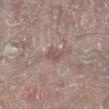{"biopsy_status": "not biopsied; imaged during a skin examination", "site": "leg", "patient": {"sex": "male", "age_approx": 65}, "lesion_size": {"long_diameter_mm_approx": 2.5}, "image": {"source": "total-body photography crop", "field_of_view_mm": 15}, "lighting": "white-light"}